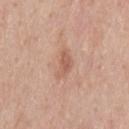{
  "biopsy_status": "not biopsied; imaged during a skin examination",
  "patient": {
    "sex": "male",
    "age_approx": 45
  },
  "image": {
    "source": "total-body photography crop",
    "field_of_view_mm": 15
  },
  "lesion_size": {
    "long_diameter_mm_approx": 3.0
  },
  "automated_metrics": {
    "area_mm2_approx": 4.5,
    "eccentricity": 0.85,
    "shape_asymmetry": 0.4,
    "border_irregularity_0_10": 4.0,
    "color_variation_0_10": 1.5,
    "peripheral_color_asymmetry": 0.5
  },
  "site": "upper back",
  "lighting": "white-light"
}